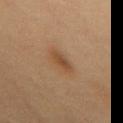This lesion was catalogued during total-body skin photography and was not selected for biopsy. A 15 mm crop from a total-body photograph taken for skin-cancer surveillance. A female patient about 80 years old. From the chest. The tile uses cross-polarized illumination.